The subject is a male aged approximately 40 · the lesion is located on the leg · cropped from a whole-body photographic skin survey; the tile spans about 15 mm:
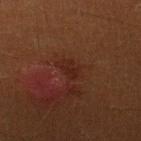Longest diameter approximately 2.5 mm. Automated image analysis of the tile measured an area of roughly 3 mm² and a shape eccentricity near 0.8. And it measured a lesion–skin lightness drop of about 4 and a normalized border contrast of about 5.5. The software also gave peripheral color asymmetry of about 0. It also reported an automated nevus-likeness rating near 5 out of 100 and a detector confidence of about 100 out of 100 that the crop contains a lesion. This is a cross-polarized tile. Histopathological examination showed a dysplastic (Clark) nevus.